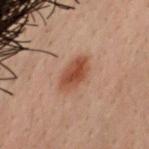| field | value |
|---|---|
| workup | total-body-photography surveillance lesion; no biopsy |
| lighting | cross-polarized illumination |
| patient | male, aged 28 to 32 |
| image source | total-body-photography crop, ~15 mm field of view |
| location | the chest |